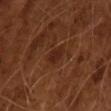Part of a total-body skin-imaging series; this lesion was reviewed on a skin check and was not flagged for biopsy.
Automated tile analysis of the lesion measured a mean CIELAB color near L≈25 a*≈21 b*≈27, about 6 CIELAB-L* units darker than the surrounding skin, and a normalized border contrast of about 7.
Imaged with cross-polarized lighting.
A region of skin cropped from a whole-body photographic capture, roughly 15 mm wide.
The lesion's longest dimension is about 2.5 mm.
A male subject aged approximately 65.